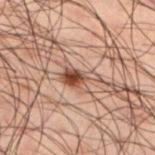follow-up: no biopsy performed (imaged during a skin exam) | patient: male, approximately 50 years of age | diameter: ≈4 mm | image: 15 mm crop, total-body photography | illumination: cross-polarized illumination | anatomic site: the left lower leg.